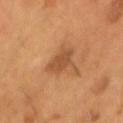Impression:
The lesion was tiled from a total-body skin photograph and was not biopsied.